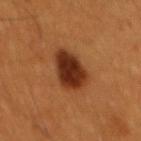The lesion is located on the mid back. A male subject roughly 60 years of age. The tile uses cross-polarized illumination. A 15 mm close-up extracted from a 3D total-body photography capture. An algorithmic analysis of the crop reported a lesion-detection confidence of about 100/100.